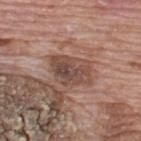Q: Was a biopsy performed?
A: catalogued during a skin exam; not biopsied
Q: What kind of image is this?
A: total-body-photography crop, ~15 mm field of view
Q: Patient demographics?
A: male, aged 68–72
Q: What is the anatomic site?
A: the upper back